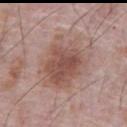follow-up = imaged on a skin check; not biopsied | image source = ~15 mm crop, total-body skin-cancer survey | patient = male, aged 68 to 72 | anatomic site = the front of the torso | illumination = white-light | image-analysis metrics = a mean CIELAB color near L≈52 a*≈20 b*≈24; border irregularity of about 4 on a 0–10 scale, internal color variation of about 5 on a 0–10 scale, and peripheral color asymmetry of about 1.5.